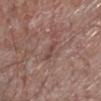Assessment: Captured during whole-body skin photography for melanoma surveillance; the lesion was not biopsied. Background: The subject is a male aged 63 to 67. From the left lower leg. A 15 mm crop from a total-body photograph taken for skin-cancer surveillance.